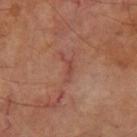site: right thigh
patient:
  sex: male
  age_approx: 70
image:
  source: total-body photography crop
  field_of_view_mm: 15